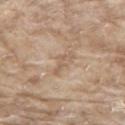biopsy status = imaged on a skin check; not biopsied | lighting = white-light | subject = male, aged approximately 65 | diameter = ~3.5 mm (longest diameter) | image source = ~15 mm crop, total-body skin-cancer survey | image-analysis metrics = an eccentricity of roughly 0.95 and two-axis asymmetry of about 0.7; a border-irregularity index near 8/10, a within-lesion color-variation index near 0/10, and radial color variation of about 0 | site = the upper back.